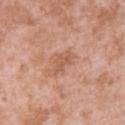The lesion was photographed on a routine skin check and not biopsied; there is no pathology result. This is a white-light tile. The lesion is on the back. The recorded lesion diameter is about 3 mm. A female patient, in their 40s. Cropped from a total-body skin-imaging series; the visible field is about 15 mm.